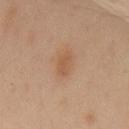Acquisition and patient details: The lesion is on the back. A 15 mm close-up tile from a total-body photography series done for melanoma screening. The patient is a male aged approximately 55.